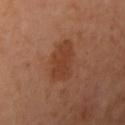workup — total-body-photography surveillance lesion; no biopsy
image-analysis metrics — a footprint of about 11 mm², an outline eccentricity of about 0.75 (0 = round, 1 = elongated), and two-axis asymmetry of about 0.25; a within-lesion color-variation index near 2/10 and radial color variation of about 0.5; a nevus-likeness score of about 40/100 and a lesion-detection confidence of about 100/100
subject — female, approximately 55 years of age
location — the right upper arm
image — ~15 mm tile from a whole-body skin photo